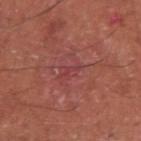Findings:
* size: about 2 mm
* anatomic site: the right upper arm
* patient: male, in their 70s
* imaging modality: total-body-photography crop, ~15 mm field of view
* diagnosis: a nodular basal cell carcinoma — a malignant lesion